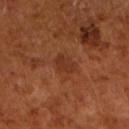The total-body-photography lesion software estimated an area of roughly 4 mm², an outline eccentricity of about 0.7 (0 = round, 1 = elongated), and a shape-asymmetry score of about 0.25 (0 = symmetric). The analysis additionally found about 6 CIELAB-L* units darker than the surrounding skin. The patient is a male aged 63–67. A close-up tile cropped from a whole-body skin photograph, about 15 mm across. The recorded lesion diameter is about 2.5 mm.